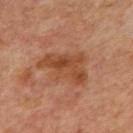Impression:
The lesion was tiled from a total-body skin photograph and was not biopsied.
Acquisition and patient details:
The subject is about 65 years old. The recorded lesion diameter is about 5.5 mm. On the back. The lesion-visualizer software estimated internal color variation of about 5 on a 0–10 scale and radial color variation of about 2. A region of skin cropped from a whole-body photographic capture, roughly 15 mm wide. The tile uses cross-polarized illumination.